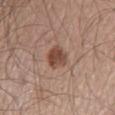Q: Is there a histopathology result?
A: no biopsy performed (imaged during a skin exam)
Q: Lesion size?
A: ~3 mm (longest diameter)
Q: Illumination type?
A: white-light
Q: Lesion location?
A: the leg
Q: What are the patient's age and sex?
A: male, about 60 years old
Q: How was this image acquired?
A: ~15 mm tile from a whole-body skin photo